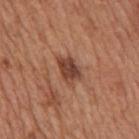Notes:
– biopsy status: total-body-photography surveillance lesion; no biopsy
– location: the mid back
– TBP lesion metrics: a nevus-likeness score of about 5/100 and lesion-presence confidence of about 100/100
– subject: male, in their mid- to late 60s
– tile lighting: white-light
– imaging modality: ~15 mm crop, total-body skin-cancer survey